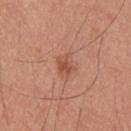Impression:
Captured during whole-body skin photography for melanoma surveillance; the lesion was not biopsied.
Context:
A male subject, roughly 30 years of age. The lesion is on the upper back. A 15 mm close-up extracted from a 3D total-body photography capture.